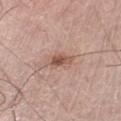Findings:
– notes: catalogued during a skin exam; not biopsied
– image: 15 mm crop, total-body photography
– subject: male, roughly 70 years of age
– body site: the right leg